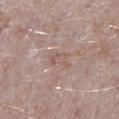Imaged during a routine full-body skin examination; the lesion was not biopsied and no histopathology is available.
Imaged with white-light lighting.
The recorded lesion diameter is about 2.5 mm.
On the left lower leg.
A lesion tile, about 15 mm wide, cut from a 3D total-body photograph.
A male subject aged 43–47.